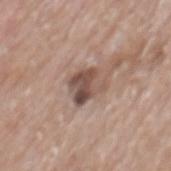workup: catalogued during a skin exam; not biopsied | subject: male, approximately 70 years of age | body site: the mid back | diameter: ≈3 mm | automated lesion analysis: an area of roughly 8 mm², an eccentricity of roughly 0.4, and a shape-asymmetry score of about 0.25 (0 = symmetric); roughly 13 lightness units darker than nearby skin and a normalized border contrast of about 9; a border-irregularity rating of about 3/10 and peripheral color asymmetry of about 2 | image source: 15 mm crop, total-body photography | tile lighting: white-light.